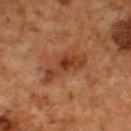Recorded during total-body skin imaging; not selected for excision or biopsy. The lesion's longest dimension is about 5.5 mm. The subject is a male in their mid- to late 60s. A 15 mm crop from a total-body photograph taken for skin-cancer surveillance. This is a cross-polarized tile. From the upper back.